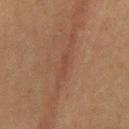Impression:
The lesion was photographed on a routine skin check and not biopsied; there is no pathology result.
Context:
A female subject, aged 58–62. Located on the mid back. The tile uses cross-polarized illumination. A lesion tile, about 15 mm wide, cut from a 3D total-body photograph. The lesion's longest dimension is about 3 mm.